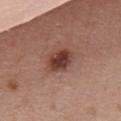| feature | finding |
|---|---|
| size | about 3.5 mm |
| image source | total-body-photography crop, ~15 mm field of view |
| subject | female, about 40 years old |
| body site | the chest |
| tile lighting | white-light illumination |
| TBP lesion metrics | a color-variation rating of about 5.5/10 and peripheral color asymmetry of about 2; a classifier nevus-likeness of about 95/100 and a detector confidence of about 100 out of 100 that the crop contains a lesion |